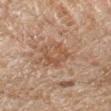Notes:
- workup · no biopsy performed (imaged during a skin exam)
- illumination · cross-polarized illumination
- subject · male, roughly 45 years of age
- site · the arm
- acquisition · ~15 mm crop, total-body skin-cancer survey
- TBP lesion metrics · a footprint of about 6.5 mm², an eccentricity of roughly 0.6, and a shape-asymmetry score of about 0.4 (0 = symmetric); a mean CIELAB color near L≈41 a*≈16 b*≈26 and a normalized lesion–skin contrast near 6